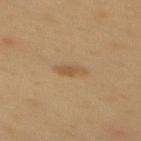Impression:
Imaged during a routine full-body skin examination; the lesion was not biopsied and no histopathology is available.
Acquisition and patient details:
Automated tile analysis of the lesion measured a classifier nevus-likeness of about 45/100 and lesion-presence confidence of about 100/100. A 15 mm close-up extracted from a 3D total-body photography capture. On the mid back. A female patient in their 50s. The tile uses cross-polarized illumination.